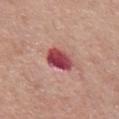| key | value |
|---|---|
| workup | catalogued during a skin exam; not biopsied |
| image source | ~15 mm crop, total-body skin-cancer survey |
| lesion size | ≈3.5 mm |
| subject | female, in their mid-60s |
| anatomic site | the chest |